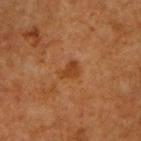Assessment:
Part of a total-body skin-imaging series; this lesion was reviewed on a skin check and was not flagged for biopsy.
Context:
Captured under cross-polarized illumination. Cropped from a total-body skin-imaging series; the visible field is about 15 mm. Located on the upper back. A male subject, aged 63–67. Measured at roughly 2.5 mm in maximum diameter. The lesion-visualizer software estimated border irregularity of about 3.5 on a 0–10 scale, a within-lesion color-variation index near 1/10, and a peripheral color-asymmetry measure near 0.5. The software also gave an automated nevus-likeness rating near 15 out of 100 and lesion-presence confidence of about 100/100.